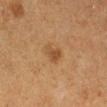workup — catalogued during a skin exam; not biopsied | size — ~2.5 mm (longest diameter) | lighting — cross-polarized | subject — female, roughly 40 years of age | image source — ~15 mm crop, total-body skin-cancer survey | image-analysis metrics — a footprint of about 4 mm², an outline eccentricity of about 0.65 (0 = round, 1 = elongated), and a symmetry-axis asymmetry near 0.35; about 7 CIELAB-L* units darker than the surrounding skin and a normalized border contrast of about 6; a border-irregularity rating of about 3/10 and radial color variation of about 0.5 | body site — the right lower leg.